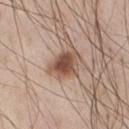Imaged during a routine full-body skin examination; the lesion was not biopsied and no histopathology is available. Automated tile analysis of the lesion measured a nevus-likeness score of about 75/100 and a detector confidence of about 100 out of 100 that the crop contains a lesion. A close-up tile cropped from a whole-body skin photograph, about 15 mm across. A male patient about 45 years old. The lesion is on the chest. Imaged with white-light lighting. Measured at roughly 2.5 mm in maximum diameter.